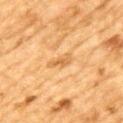– workup · total-body-photography surveillance lesion; no biopsy
– patient · male, aged approximately 85 or older
– body site · the mid back
– automated metrics · a lesion area of about 4 mm² and a shape eccentricity near 0.9; border irregularity of about 4 on a 0–10 scale and internal color variation of about 1.5 on a 0–10 scale; a lesion-detection confidence of about 80/100
– image · total-body-photography crop, ~15 mm field of view
– lesion size · ≈3.5 mm